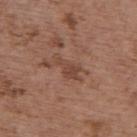workup: total-body-photography surveillance lesion; no biopsy
patient: female, in their mid-60s
anatomic site: the upper back
illumination: white-light
lesion diameter: about 4 mm
automated lesion analysis: roughly 7 lightness units darker than nearby skin and a normalized border contrast of about 6
image: ~15 mm tile from a whole-body skin photo